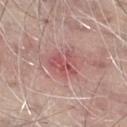Part of a total-body skin-imaging series; this lesion was reviewed on a skin check and was not flagged for biopsy. The total-body-photography lesion software estimated a border-irregularity index near 6.5/10 and radial color variation of about 0.5. The analysis additionally found a detector confidence of about 100 out of 100 that the crop contains a lesion. Located on the right thigh. A region of skin cropped from a whole-body photographic capture, roughly 15 mm wide. The recorded lesion diameter is about 3.5 mm. The patient is a male in their mid- to late 60s. Imaged with white-light lighting.